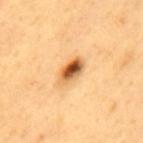Case summary:
* follow-up — catalogued during a skin exam; not biopsied
* TBP lesion metrics — a footprint of about 6.5 mm², a shape eccentricity near 0.85, and a symmetry-axis asymmetry near 0.2; a mean CIELAB color near L≈61 a*≈24 b*≈45 and a lesion–skin lightness drop of about 18; an automated nevus-likeness rating near 100 out of 100 and a lesion-detection confidence of about 100/100
* diameter — about 4 mm
* image — ~15 mm crop, total-body skin-cancer survey
* body site — the mid back
* illumination — cross-polarized
* subject — male, roughly 60 years of age